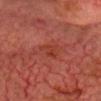No biopsy was performed on this lesion — it was imaged during a full skin examination and was not determined to be concerning. The lesion is located on the head or neck. Longest diameter approximately 3 mm. A roughly 15 mm field-of-view crop from a total-body skin photograph. The lesion-visualizer software estimated a border-irregularity rating of about 4.5/10 and internal color variation of about 2.5 on a 0–10 scale. Captured under cross-polarized illumination. A male subject, aged approximately 75.